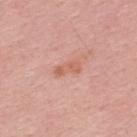Findings:
• notes: imaged on a skin check; not biopsied
• image: ~15 mm crop, total-body skin-cancer survey
• body site: the upper back
• subject: male, roughly 30 years of age
• lesion diameter: ≈3 mm
• TBP lesion metrics: an outline eccentricity of about 0.9 (0 = round, 1 = elongated) and a symmetry-axis asymmetry near 0.3; a mean CIELAB color near L≈61 a*≈26 b*≈30, roughly 8 lightness units darker than nearby skin, and a lesion-to-skin contrast of about 6 (normalized; higher = more distinct)